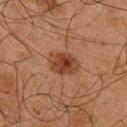automated_metrics:
  color_variation_0_10: 4.0
  peripheral_color_asymmetry: 1.5
lighting: cross-polarized
site: right lower leg
patient:
  sex: male
  age_approx: 65
image:
  source: total-body photography crop
  field_of_view_mm: 15
lesion_size:
  long_diameter_mm_approx: 3.5